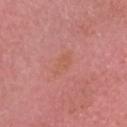Q: Was this lesion biopsied?
A: total-body-photography surveillance lesion; no biopsy
Q: How was this image acquired?
A: total-body-photography crop, ~15 mm field of view
Q: Lesion location?
A: the head or neck
Q: Illumination type?
A: white-light
Q: Patient demographics?
A: male, aged 68 to 72
Q: How large is the lesion?
A: ≈3 mm